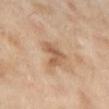Imaged during a routine full-body skin examination; the lesion was not biopsied and no histopathology is available.
A female subject, in their 70s.
An algorithmic analysis of the crop reported a lesion color around L≈59 a*≈19 b*≈33 in CIELAB and a lesion–skin lightness drop of about 10. The software also gave a within-lesion color-variation index near 3/10 and peripheral color asymmetry of about 1. The software also gave an automated nevus-likeness rating near 0 out of 100 and a lesion-detection confidence of about 100/100.
Cropped from a total-body skin-imaging series; the visible field is about 15 mm.
Longest diameter approximately 3 mm.
Located on the left lower leg.
This is a cross-polarized tile.